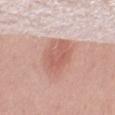Imaged during a routine full-body skin examination; the lesion was not biopsied and no histopathology is available. The lesion-visualizer software estimated a mean CIELAB color near L≈61 a*≈24 b*≈27, a lesion–skin lightness drop of about 10, and a lesion-to-skin contrast of about 6.5 (normalized; higher = more distinct). It also reported a border-irregularity rating of about 2/10, internal color variation of about 3 on a 0–10 scale, and a peripheral color-asymmetry measure near 1. A female subject, in their 60s. The lesion is located on the right thigh. Imaged with white-light lighting. This image is a 15 mm lesion crop taken from a total-body photograph.